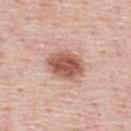biopsy status: total-body-photography surveillance lesion; no biopsy | subject: male, roughly 45 years of age | image source: ~15 mm crop, total-body skin-cancer survey | lighting: white-light illumination | site: the mid back | size: ~4.5 mm (longest diameter) | automated lesion analysis: a shape eccentricity near 0.65 and a shape-asymmetry score of about 0.15 (0 = symmetric); border irregularity of about 1.5 on a 0–10 scale, a within-lesion color-variation index near 5/10, and peripheral color asymmetry of about 2; an automated nevus-likeness rating near 100 out of 100 and a lesion-detection confidence of about 100/100.